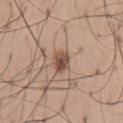notes: no biopsy performed (imaged during a skin exam) | imaging modality: ~15 mm crop, total-body skin-cancer survey | subject: male, aged approximately 65 | body site: the lower back.